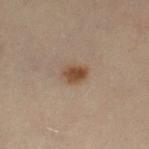Q: Is there a histopathology result?
A: no biopsy performed (imaged during a skin exam)
Q: What is the imaging modality?
A: total-body-photography crop, ~15 mm field of view
Q: Lesion location?
A: the left thigh
Q: Patient demographics?
A: female, in their 30s